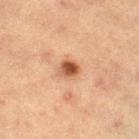Assessment: Captured during whole-body skin photography for melanoma surveillance; the lesion was not biopsied. Context: Longest diameter approximately 2.5 mm. A female subject about 55 years old. On the right thigh. Captured under cross-polarized illumination. Automated tile analysis of the lesion measured an area of roughly 4.5 mm², an eccentricity of roughly 0.5, and a shape-asymmetry score of about 0.2 (0 = symmetric). Cropped from a whole-body photographic skin survey; the tile spans about 15 mm.